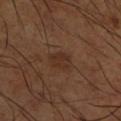Imaged during a routine full-body skin examination; the lesion was not biopsied and no histopathology is available.